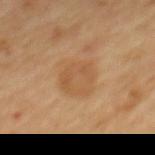The lesion was tiled from a total-body skin photograph and was not biopsied.